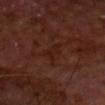No biopsy was performed on this lesion — it was imaged during a full skin examination and was not determined to be concerning. The subject is a male aged 68–72. About 3 mm across. The total-body-photography lesion software estimated a footprint of about 3.5 mm² and a symmetry-axis asymmetry near 0.4. It also reported a border-irregularity index near 4.5/10 and internal color variation of about 0 on a 0–10 scale. A 15 mm close-up tile from a total-body photography series done for melanoma screening. Captured under cross-polarized illumination. Located on the chest.